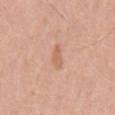workup — imaged on a skin check; not biopsied
imaging modality — ~15 mm crop, total-body skin-cancer survey
patient — male, aged 43 to 47
lighting — white-light
image-analysis metrics — a footprint of about 4 mm², a shape eccentricity near 0.85, and a symmetry-axis asymmetry near 0.25; an average lesion color of about L≈63 a*≈22 b*≈33 (CIELAB), a lesion–skin lightness drop of about 7, and a normalized lesion–skin contrast near 5
anatomic site — the front of the torso